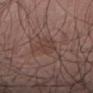{
  "biopsy_status": "not biopsied; imaged during a skin examination",
  "lighting": "white-light",
  "patient": {
    "sex": "male",
    "age_approx": 70
  },
  "site": "right thigh",
  "image": {
    "source": "total-body photography crop",
    "field_of_view_mm": 15
  }
}